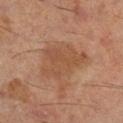A region of skin cropped from a whole-body photographic capture, roughly 15 mm wide. Captured under cross-polarized illumination. The patient is a male aged approximately 60. The lesion is located on the right lower leg. Automated tile analysis of the lesion measured border irregularity of about 5 on a 0–10 scale, a within-lesion color-variation index near 3/10, and a peripheral color-asymmetry measure near 1. And it measured a classifier nevus-likeness of about 0/100 and a lesion-detection confidence of about 100/100. About 5.5 mm across.